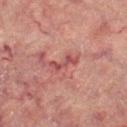biopsy_status: not biopsied; imaged during a skin examination
site: right lower leg
lighting: cross-polarized
lesion_size:
  long_diameter_mm_approx: 3.5
image:
  source: total-body photography crop
  field_of_view_mm: 15
patient:
  sex: female
  age_approx: 70
automated_metrics:
  area_mm2_approx: 4.0
  eccentricity: 0.95
  shape_asymmetry: 0.5
  nevus_likeness_0_100: 0
  lesion_detection_confidence_0_100: 90